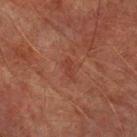Notes:
• follow-up — imaged on a skin check; not biopsied
• acquisition — ~15 mm crop, total-body skin-cancer survey
• tile lighting — cross-polarized illumination
• size — ≈2.5 mm
• subject — male, in their mid-70s
• automated lesion analysis — a lesion–skin lightness drop of about 5 and a lesion-to-skin contrast of about 5 (normalized; higher = more distinct); an automated nevus-likeness rating near 0 out of 100 and a detector confidence of about 100 out of 100 that the crop contains a lesion
• site — the left forearm